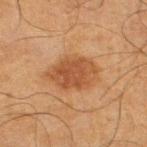The lesion was photographed on a routine skin check and not biopsied; there is no pathology result.
A male patient, aged around 65.
From the leg.
Cropped from a whole-body photographic skin survey; the tile spans about 15 mm.